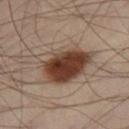follow-up — total-body-photography surveillance lesion; no biopsy | imaging modality — ~15 mm crop, total-body skin-cancer survey | lesion diameter — ≈7 mm | body site — the right thigh | tile lighting — cross-polarized illumination | subject — male, about 40 years old.